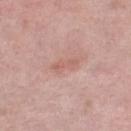Impression: No biopsy was performed on this lesion — it was imaged during a full skin examination and was not determined to be concerning. Acquisition and patient details: A female patient, in their 60s. Imaged with white-light lighting. The lesion is located on the right lower leg. A close-up tile cropped from a whole-body skin photograph, about 15 mm across. The lesion-visualizer software estimated an area of roughly 3.5 mm², an eccentricity of roughly 0.85, and a shape-asymmetry score of about 0.3 (0 = symmetric). And it measured a lesion color around L≈60 a*≈23 b*≈26 in CIELAB and a lesion-to-skin contrast of about 5 (normalized; higher = more distinct). The analysis additionally found an automated nevus-likeness rating near 0 out of 100 and a detector confidence of about 100 out of 100 that the crop contains a lesion. About 3 mm across.